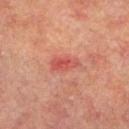Clinical impression: The lesion was photographed on a routine skin check and not biopsied; there is no pathology result. Background: On the left lower leg. The total-body-photography lesion software estimated a footprint of about 5 mm², a shape eccentricity near 0.9, and a shape-asymmetry score of about 0.3 (0 = symmetric). The software also gave border irregularity of about 3.5 on a 0–10 scale, internal color variation of about 2.5 on a 0–10 scale, and peripheral color asymmetry of about 1. The software also gave an automated nevus-likeness rating near 0 out of 100. This is a cross-polarized tile. A male subject aged 63–67. A 15 mm close-up extracted from a 3D total-body photography capture. Measured at roughly 3.5 mm in maximum diameter.